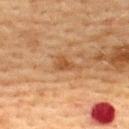Impression:
Imaged during a routine full-body skin examination; the lesion was not biopsied and no histopathology is available.
Clinical summary:
About 3 mm across. A lesion tile, about 15 mm wide, cut from a 3D total-body photograph. This is a cross-polarized tile. The lesion is located on the upper back. A female subject roughly 55 years of age.